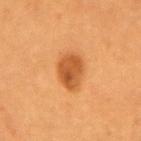This lesion was catalogued during total-body skin photography and was not selected for biopsy. Located on the left upper arm. Captured under cross-polarized illumination. A female patient, aged around 55. A region of skin cropped from a whole-body photographic capture, roughly 15 mm wide.